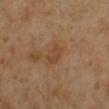Captured during whole-body skin photography for melanoma surveillance; the lesion was not biopsied.
The lesion-visualizer software estimated a lesion area of about 4.5 mm² and a symmetry-axis asymmetry near 0.2. The analysis additionally found a border-irregularity rating of about 2/10, a color-variation rating of about 1/10, and peripheral color asymmetry of about 0.5. It also reported a classifier nevus-likeness of about 0/100.
Imaged with cross-polarized lighting.
Approximately 3 mm at its widest.
The patient is a female in their 50s.
The lesion is on the right lower leg.
A roughly 15 mm field-of-view crop from a total-body skin photograph.